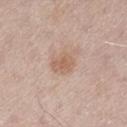Clinical impression:
The lesion was photographed on a routine skin check and not biopsied; there is no pathology result.
Acquisition and patient details:
On the left thigh. The recorded lesion diameter is about 3 mm. Automated image analysis of the tile measured a shape eccentricity near 0.5 and two-axis asymmetry of about 0.2. It also reported an average lesion color of about L≈60 a*≈18 b*≈29 (CIELAB) and about 8 CIELAB-L* units darker than the surrounding skin. And it measured a border-irregularity index near 1.5/10 and a color-variation rating of about 2.5/10. The analysis additionally found a classifier nevus-likeness of about 45/100 and lesion-presence confidence of about 100/100. A male patient, aged 63 to 67. Imaged with white-light lighting. A close-up tile cropped from a whole-body skin photograph, about 15 mm across.